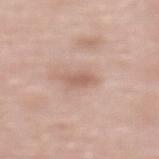Clinical impression:
The lesion was photographed on a routine skin check and not biopsied; there is no pathology result.
Acquisition and patient details:
A roughly 15 mm field-of-view crop from a total-body skin photograph. Captured under white-light illumination. From the chest. A female patient aged 63 to 67. Longest diameter approximately 3 mm.